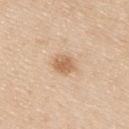Q: Is there a histopathology result?
A: no biopsy performed (imaged during a skin exam)
Q: What are the patient's age and sex?
A: male, in their mid- to late 30s
Q: Where on the body is the lesion?
A: the back
Q: What kind of image is this?
A: total-body-photography crop, ~15 mm field of view
Q: How was the tile lit?
A: white-light illumination
Q: Lesion size?
A: ≈2.5 mm